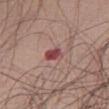<tbp_lesion>
<biopsy_status>not biopsied; imaged during a skin examination</biopsy_status>
<lighting>white-light</lighting>
<automated_metrics>
  <border_irregularity_0_10>3.0</border_irregularity_0_10>
  <color_variation_0_10>5.0</color_variation_0_10>
  <peripheral_color_asymmetry>2.0</peripheral_color_asymmetry>
  <nevus_likeness_0_100>5</nevus_likeness_0_100>
  <lesion_detection_confidence_0_100>100</lesion_detection_confidence_0_100>
</automated_metrics>
<image>
  <source>total-body photography crop</source>
  <field_of_view_mm>15</field_of_view_mm>
</image>
<patient>
  <sex>male</sex>
  <age_approx>75</age_approx>
</patient>
<site>abdomen</site>
<lesion_size>
  <long_diameter_mm_approx>2.5</long_diameter_mm_approx>
</lesion_size>
</tbp_lesion>